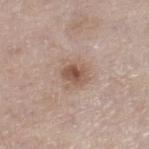| feature | finding |
|---|---|
| workup | total-body-photography surveillance lesion; no biopsy |
| location | the right lower leg |
| patient | male, aged 78–82 |
| image | 15 mm crop, total-body photography |
| lesion size | ≈3 mm |
| tile lighting | white-light illumination |